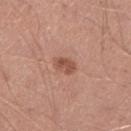This lesion was catalogued during total-body skin photography and was not selected for biopsy. A male subject aged 43–47. About 3 mm across. The lesion is on the right lower leg. A roughly 15 mm field-of-view crop from a total-body skin photograph. Captured under white-light illumination.